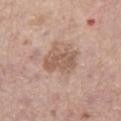Clinical impression: Part of a total-body skin-imaging series; this lesion was reviewed on a skin check and was not flagged for biopsy. Background: This image is a 15 mm lesion crop taken from a total-body photograph. Located on the right thigh. A male patient aged approximately 75.